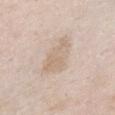Background:
A female subject aged around 75. A region of skin cropped from a whole-body photographic capture, roughly 15 mm wide. On the chest.